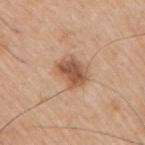Q: Is there a histopathology result?
A: catalogued during a skin exam; not biopsied
Q: Lesion location?
A: the right upper arm
Q: Who is the patient?
A: male, about 75 years old
Q: How was this image acquired?
A: total-body-photography crop, ~15 mm field of view